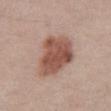notes: catalogued during a skin exam; not biopsied
site: the abdomen
image source: total-body-photography crop, ~15 mm field of view
patient: male, aged 38–42
tile lighting: white-light illumination